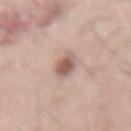{
  "image": {
    "source": "total-body photography crop",
    "field_of_view_mm": 15
  },
  "lighting": "white-light",
  "patient": {
    "sex": "male",
    "age_approx": 60
  },
  "site": "arm",
  "lesion_size": {
    "long_diameter_mm_approx": 3.0
  }
}